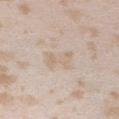{"biopsy_status": "not biopsied; imaged during a skin examination", "patient": {"sex": "female", "age_approx": 25}, "lighting": "white-light", "lesion_size": {"long_diameter_mm_approx": 3.5}, "site": "right upper arm", "image": {"source": "total-body photography crop", "field_of_view_mm": 15}}